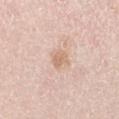{"biopsy_status": "not biopsied; imaged during a skin examination", "image": {"source": "total-body photography crop", "field_of_view_mm": 15}, "site": "left upper arm", "patient": {"sex": "male", "age_approx": 45}}